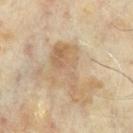Captured during whole-body skin photography for melanoma surveillance; the lesion was not biopsied.
Cropped from a total-body skin-imaging series; the visible field is about 15 mm.
The subject is a male aged around 65.
From the chest.
Imaged with cross-polarized lighting.